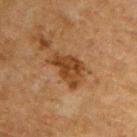Clinical impression:
The lesion was tiled from a total-body skin photograph and was not biopsied.
Image and clinical context:
A male patient, in their mid- to late 60s. Approximately 4.5 mm at its widest. Captured under cross-polarized illumination. An algorithmic analysis of the crop reported an area of roughly 10 mm² and an eccentricity of roughly 0.75. The software also gave a lesion color around L≈39 a*≈21 b*≈35 in CIELAB, a lesion–skin lightness drop of about 10, and a lesion-to-skin contrast of about 9 (normalized; higher = more distinct). The analysis additionally found a border-irregularity rating of about 4.5/10 and radial color variation of about 1.5. The software also gave an automated nevus-likeness rating near 10 out of 100 and lesion-presence confidence of about 100/100. The lesion is on the upper back. A roughly 15 mm field-of-view crop from a total-body skin photograph.